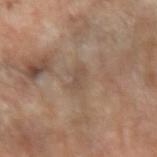Q: Was this lesion biopsied?
A: imaged on a skin check; not biopsied
Q: What is the lesion's diameter?
A: ~2.5 mm (longest diameter)
Q: Automated lesion metrics?
A: a footprint of about 3 mm²; about 7 CIELAB-L* units darker than the surrounding skin and a normalized border contrast of about 5; lesion-presence confidence of about 95/100
Q: What is the imaging modality?
A: 15 mm crop, total-body photography
Q: Lesion location?
A: the left forearm
Q: Patient demographics?
A: female, roughly 70 years of age